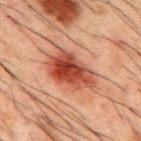Recorded during total-body skin imaging; not selected for excision or biopsy.
The lesion is on the mid back.
Longest diameter approximately 6 mm.
A male patient about 50 years old.
This is a cross-polarized tile.
Cropped from a total-body skin-imaging series; the visible field is about 15 mm.